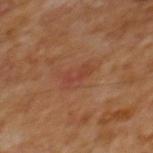Notes:
• biopsy status — no biopsy performed (imaged during a skin exam)
• subject — male, approximately 65 years of age
• location — the mid back
• acquisition — 15 mm crop, total-body photography